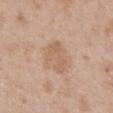Clinical impression: The lesion was tiled from a total-body skin photograph and was not biopsied. Acquisition and patient details: A female patient in their 30s. Located on the mid back. A region of skin cropped from a whole-body photographic capture, roughly 15 mm wide.